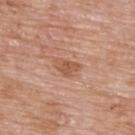Imaged during a routine full-body skin examination; the lesion was not biopsied and no histopathology is available.